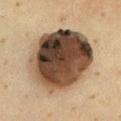Captured during whole-body skin photography for melanoma surveillance; the lesion was not biopsied. The recorded lesion diameter is about 8.5 mm. A female patient aged around 40. The tile uses cross-polarized illumination. A roughly 15 mm field-of-view crop from a total-body skin photograph. Located on the chest.